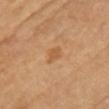<tbp_lesion>
  <biopsy_status>not biopsied; imaged during a skin examination</biopsy_status>
  <lesion_size>
    <long_diameter_mm_approx>2.5</long_diameter_mm_approx>
  </lesion_size>
  <patient>
    <sex>female</sex>
    <age_approx>65</age_approx>
  </patient>
  <image>
    <source>total-body photography crop</source>
    <field_of_view_mm>15</field_of_view_mm>
  </image>
  <site>chest</site>
</tbp_lesion>